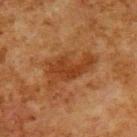{
  "biopsy_status": "not biopsied; imaged during a skin examination",
  "lesion_size": {
    "long_diameter_mm_approx": 6.5
  },
  "patient": {
    "sex": "male",
    "age_approx": 80
  },
  "image": {
    "source": "total-body photography crop",
    "field_of_view_mm": 15
  },
  "automated_metrics": {
    "eccentricity": 0.9,
    "shape_asymmetry": 0.4,
    "vs_skin_darker_L": 8.0,
    "vs_skin_contrast_norm": 7.5
  },
  "lighting": "cross-polarized",
  "site": "upper back"
}